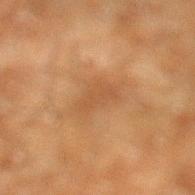Clinical summary: Imaged with cross-polarized lighting. A male patient aged approximately 60. On the leg. A region of skin cropped from a whole-body photographic capture, roughly 15 mm wide.